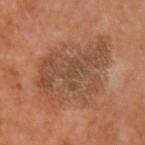  biopsy_status: not biopsied; imaged during a skin examination
  lighting: cross-polarized
  lesion_size:
    long_diameter_mm_approx: 8.0
  site: left upper arm
  patient:
    sex: male
    age_approx: 55
  image:
    source: total-body photography crop
    field_of_view_mm: 15
  automated_metrics:
    area_mm2_approx: 29.0
    eccentricity: 0.8
    shape_asymmetry: 0.4
    border_irregularity_0_10: 7.5
    color_variation_0_10: 4.5
    nevus_likeness_0_100: 0
    lesion_detection_confidence_0_100: 100